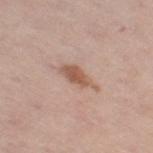{"biopsy_status": "not biopsied; imaged during a skin examination", "lighting": "white-light", "patient": {"sex": "female", "age_approx": 65}, "site": "right thigh", "image": {"source": "total-body photography crop", "field_of_view_mm": 15}, "automated_metrics": {"cielab_L": 56, "cielab_a": 20, "cielab_b": 29, "vs_skin_darker_L": 11.0, "vs_skin_contrast_norm": 8.0}}